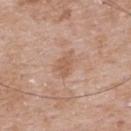| key | value |
|---|---|
| biopsy status | imaged on a skin check; not biopsied |
| lighting | white-light illumination |
| patient | male, aged approximately 50 |
| acquisition | ~15 mm crop, total-body skin-cancer survey |
| body site | the mid back |
| diameter | ~2.5 mm (longest diameter) |